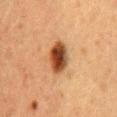Assessment:
The lesion was photographed on a routine skin check and not biopsied; there is no pathology result.
Image and clinical context:
Located on the lower back. A female subject in their 50s. Imaged with cross-polarized lighting. Measured at roughly 4.5 mm in maximum diameter. Automated image analysis of the tile measured a footprint of about 10 mm², a shape eccentricity near 0.8, and a symmetry-axis asymmetry near 0.1. And it measured an average lesion color of about L≈40 a*≈21 b*≈32 (CIELAB), a lesion–skin lightness drop of about 16, and a normalized lesion–skin contrast near 12. A 15 mm close-up tile from a total-body photography series done for melanoma screening.